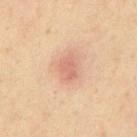Findings:
• workup — catalogued during a skin exam; not biopsied
• lesion size — ~3 mm (longest diameter)
• body site — the abdomen
• image — ~15 mm tile from a whole-body skin photo
• image-analysis metrics — a footprint of about 5 mm², an outline eccentricity of about 0.75 (0 = round, 1 = elongated), and two-axis asymmetry of about 0.25; a lesion color around L≈64 a*≈24 b*≈30 in CIELAB, about 9 CIELAB-L* units darker than the surrounding skin, and a normalized lesion–skin contrast near 6; border irregularity of about 2.5 on a 0–10 scale, a color-variation rating of about 1.5/10, and a peripheral color-asymmetry measure near 0.5
• tile lighting — cross-polarized
• subject — male, aged 53–57